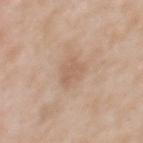Recorded during total-body skin imaging; not selected for excision or biopsy.